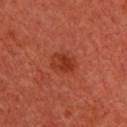biopsy status = total-body-photography surveillance lesion; no biopsy
illumination = cross-polarized illumination
patient = female, aged approximately 50
image = 15 mm crop, total-body photography